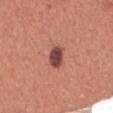Notes:
• notes · catalogued during a skin exam; not biopsied
• acquisition · ~15 mm crop, total-body skin-cancer survey
• subject · male, aged 43–47
• body site · the front of the torso
• tile lighting · white-light illumination
• lesion diameter · ≈3 mm